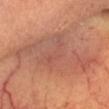The lesion-visualizer software estimated a lesion color around L≈52 a*≈24 b*≈28 in CIELAB, a lesion–skin lightness drop of about 7, and a normalized lesion–skin contrast near 6.
The lesion is located on the head or neck.
Cropped from a total-body skin-imaging series; the visible field is about 15 mm.
Captured under cross-polarized illumination.
A male subject in their 70s.